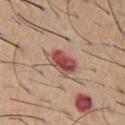Image and clinical context: Cropped from a whole-body photographic skin survey; the tile spans about 15 mm. A male subject, roughly 60 years of age. The lesion is on the chest.